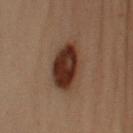lighting: cross-polarized | imaging modality: ~15 mm crop, total-body skin-cancer survey | anatomic site: the left upper arm | patient: male, aged 53 to 57 | automated lesion analysis: an average lesion color of about L≈26 a*≈16 b*≈22 (CIELAB) and a lesion-to-skin contrast of about 12.5 (normalized; higher = more distinct); border irregularity of about 1.5 on a 0–10 scale, internal color variation of about 5 on a 0–10 scale, and a peripheral color-asymmetry measure near 1.5; an automated nevus-likeness rating near 100 out of 100 and lesion-presence confidence of about 100/100.